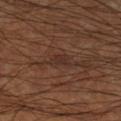Q: Was a biopsy performed?
A: catalogued during a skin exam; not biopsied
Q: What are the patient's age and sex?
A: male, aged 58–62
Q: How was this image acquired?
A: ~15 mm tile from a whole-body skin photo
Q: What did automated image analysis measure?
A: internal color variation of about 1 on a 0–10 scale; a lesion-detection confidence of about 80/100
Q: What lighting was used for the tile?
A: cross-polarized
Q: Where on the body is the lesion?
A: the left lower leg